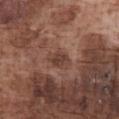Captured during whole-body skin photography for melanoma surveillance; the lesion was not biopsied. The total-body-photography lesion software estimated an area of roughly 5 mm², an outline eccentricity of about 0.7 (0 = round, 1 = elongated), and two-axis asymmetry of about 0.25. And it measured a lesion color around L≈41 a*≈19 b*≈25 in CIELAB and roughly 8 lightness units darker than nearby skin. And it measured a nevus-likeness score of about 20/100 and lesion-presence confidence of about 100/100. The subject is a male roughly 75 years of age. A lesion tile, about 15 mm wide, cut from a 3D total-body photograph. The tile uses white-light illumination. Located on the chest. The recorded lesion diameter is about 3 mm.